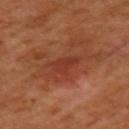Findings:
* notes: catalogued during a skin exam; not biopsied
* lesion diameter: about 3 mm
* location: the left upper arm
* image source: ~15 mm tile from a whole-body skin photo
* automated metrics: roughly 6 lightness units darker than nearby skin and a lesion-to-skin contrast of about 5.5 (normalized; higher = more distinct); a border-irregularity index near 4/10; a detector confidence of about 100 out of 100 that the crop contains a lesion
* patient: female, aged 53–57